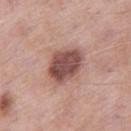Notes:
- follow-up · no biopsy performed (imaged during a skin exam)
- patient · male, in their mid- to late 50s
- body site · the right thigh
- TBP lesion metrics · a lesion area of about 13 mm², an outline eccentricity of about 0.65 (0 = round, 1 = elongated), and a shape-asymmetry score of about 0.2 (0 = symmetric); a lesion–skin lightness drop of about 16 and a lesion-to-skin contrast of about 11 (normalized; higher = more distinct); a color-variation rating of about 4/10 and radial color variation of about 1; a classifier nevus-likeness of about 70/100 and a detector confidence of about 100 out of 100 that the crop contains a lesion
- lighting · white-light
- image source · 15 mm crop, total-body photography
- lesion diameter · ~5 mm (longest diameter)